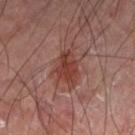{
  "biopsy_status": "not biopsied; imaged during a skin examination",
  "patient": {
    "sex": "male",
    "age_approx": 60
  },
  "lighting": "cross-polarized",
  "lesion_size": {
    "long_diameter_mm_approx": 4.0
  },
  "site": "arm",
  "image": {
    "source": "total-body photography crop",
    "field_of_view_mm": 15
  }
}